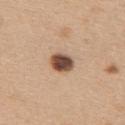This lesion was catalogued during total-body skin photography and was not selected for biopsy. On the upper back. Cropped from a whole-body photographic skin survey; the tile spans about 15 mm. A female patient in their mid-40s. An algorithmic analysis of the crop reported an area of roughly 6.5 mm², an outline eccentricity of about 0.5 (0 = round, 1 = elongated), and two-axis asymmetry of about 0.1. And it measured a mean CIELAB color near L≈49 a*≈19 b*≈28, a lesion–skin lightness drop of about 20, and a lesion-to-skin contrast of about 13 (normalized; higher = more distinct). The analysis additionally found border irregularity of about 1 on a 0–10 scale, a color-variation rating of about 6/10, and a peripheral color-asymmetry measure near 1.5.